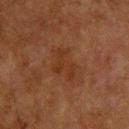size: about 4 mm | location: the upper back | patient: male, in their mid-60s | image source: total-body-photography crop, ~15 mm field of view.